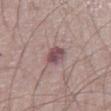Case summary:
* follow-up: total-body-photography surveillance lesion; no biopsy
* patient: male, aged 68–72
* illumination: white-light illumination
* anatomic site: the leg
* image: ~15 mm crop, total-body skin-cancer survey